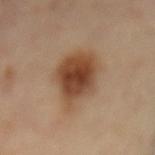follow-up: imaged on a skin check; not biopsied
illumination: cross-polarized illumination
imaging modality: ~15 mm tile from a whole-body skin photo
site: the mid back